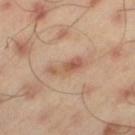Impression: Imaged during a routine full-body skin examination; the lesion was not biopsied and no histopathology is available. Image and clinical context: A male subject about 45 years old. Located on the left thigh. A 15 mm crop from a total-body photograph taken for skin-cancer surveillance. The lesion's longest dimension is about 4.5 mm. The lesion-visualizer software estimated a lesion color around L≈56 a*≈20 b*≈30 in CIELAB, a lesion–skin lightness drop of about 9, and a lesion-to-skin contrast of about 6 (normalized; higher = more distinct).